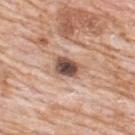Acquisition and patient details:
The subject is a male approximately 80 years of age. Captured under white-light illumination. Measured at roughly 3.5 mm in maximum diameter. Cropped from a total-body skin-imaging series; the visible field is about 15 mm. From the upper back.